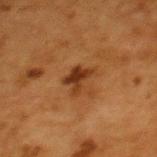Findings:
– notes — total-body-photography surveillance lesion; no biopsy
– tile lighting — cross-polarized illumination
– anatomic site — the upper back
– lesion diameter — about 3 mm
– patient — female, aged approximately 50
– image source — total-body-photography crop, ~15 mm field of view